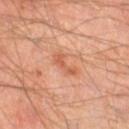Impression: Part of a total-body skin-imaging series; this lesion was reviewed on a skin check and was not flagged for biopsy. Background: A male patient roughly 45 years of age. Imaged with cross-polarized lighting. A roughly 15 mm field-of-view crop from a total-body skin photograph. Located on the leg. The lesion's longest dimension is about 3.5 mm.